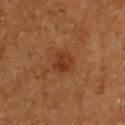{
  "biopsy_status": "not biopsied; imaged during a skin examination",
  "site": "upper back",
  "patient": {
    "sex": "female",
    "age_approx": 55
  },
  "automated_metrics": {
    "area_mm2_approx": 5.0,
    "shape_asymmetry": 0.2,
    "cielab_L": 37,
    "cielab_a": 26,
    "cielab_b": 35,
    "vs_skin_darker_L": 8.0,
    "vs_skin_contrast_norm": 6.5,
    "nevus_likeness_0_100": 55,
    "lesion_detection_confidence_0_100": 100
  },
  "image": {
    "source": "total-body photography crop",
    "field_of_view_mm": 15
  }
}